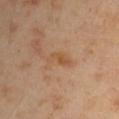Part of a total-body skin-imaging series; this lesion was reviewed on a skin check and was not flagged for biopsy. A male patient, about 45 years old. A close-up tile cropped from a whole-body skin photograph, about 15 mm across. Measured at roughly 3 mm in maximum diameter. An algorithmic analysis of the crop reported a mean CIELAB color near L≈51 a*≈20 b*≈35 and roughly 7 lightness units darker than nearby skin. The lesion is on the left upper arm.